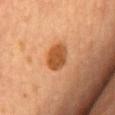| feature | finding |
|---|---|
| notes | total-body-photography surveillance lesion; no biopsy |
| location | the chest |
| automated metrics | an eccentricity of roughly 0.65 and two-axis asymmetry of about 0.2; a border-irregularity rating of about 1.5/10 |
| tile lighting | cross-polarized |
| patient | female, aged around 60 |
| image | ~15 mm crop, total-body skin-cancer survey |